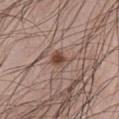A male patient approximately 25 years of age. Located on the chest. A 15 mm close-up extracted from a 3D total-body photography capture.